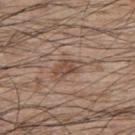imaging modality=total-body-photography crop, ~15 mm field of view | diameter=about 3.5 mm | body site=the upper back | patient=male, roughly 70 years of age | tile lighting=white-light illumination.